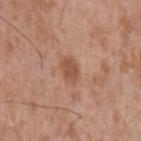<case>
  <biopsy_status>not biopsied; imaged during a skin examination</biopsy_status>
  <lesion_size>
    <long_diameter_mm_approx>2.5</long_diameter_mm_approx>
  </lesion_size>
  <lighting>white-light</lighting>
  <image>
    <source>total-body photography crop</source>
    <field_of_view_mm>15</field_of_view_mm>
  </image>
  <site>left upper arm</site>
  <patient>
    <sex>male</sex>
    <age_approx>50</age_approx>
  </patient>
</case>